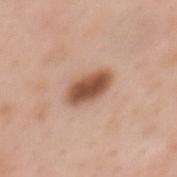The lesion was tiled from a total-body skin photograph and was not biopsied.
Imaged with white-light lighting.
The lesion is on the mid back.
Longest diameter approximately 4.5 mm.
A region of skin cropped from a whole-body photographic capture, roughly 15 mm wide.
A female patient, aged around 50.
The total-body-photography lesion software estimated about 16 CIELAB-L* units darker than the surrounding skin and a normalized lesion–skin contrast near 11. And it measured a border-irregularity rating of about 2/10, a within-lesion color-variation index near 4.5/10, and a peripheral color-asymmetry measure near 1.5.